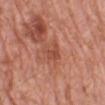{"biopsy_status": "not biopsied; imaged during a skin examination", "lesion_size": {"long_diameter_mm_approx": 3.0}, "lighting": "white-light", "site": "left lower leg", "image": {"source": "total-body photography crop", "field_of_view_mm": 15}, "patient": {"sex": "male", "age_approx": 80}}